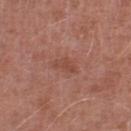This lesion was catalogued during total-body skin photography and was not selected for biopsy.
Cropped from a total-body skin-imaging series; the visible field is about 15 mm.
From the left lower leg.
A male patient, in their mid-60s.
About 3 mm across.